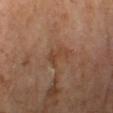Longest diameter approximately 3 mm.
Automated tile analysis of the lesion measured a lesion area of about 4 mm², an eccentricity of roughly 0.8, and a shape-asymmetry score of about 0.6 (0 = symmetric). It also reported an average lesion color of about L≈41 a*≈20 b*≈30 (CIELAB) and a normalized border contrast of about 6.
The patient is a male in their mid-60s.
A 15 mm crop from a total-body photograph taken for skin-cancer surveillance.
This is a cross-polarized tile.